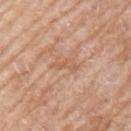Clinical impression: The lesion was tiled from a total-body skin photograph and was not biopsied. Image and clinical context: A roughly 15 mm field-of-view crop from a total-body skin photograph. The lesion is located on the left upper arm. Automated tile analysis of the lesion measured an average lesion color of about L≈59 a*≈22 b*≈33 (CIELAB), a lesion–skin lightness drop of about 7, and a normalized border contrast of about 5. The tile uses white-light illumination. A female patient in their mid- to late 70s. The lesion's longest dimension is about 3 mm.